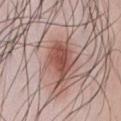Findings:
• image source — 15 mm crop, total-body photography
• patient — male, about 35 years old
• anatomic site — the chest